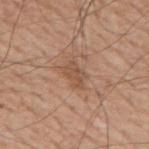Imaged during a routine full-body skin examination; the lesion was not biopsied and no histopathology is available. From the mid back. The tile uses white-light illumination. The lesion-visualizer software estimated a footprint of about 5 mm² and a shape eccentricity near 0.8. And it measured a classifier nevus-likeness of about 0/100 and a lesion-detection confidence of about 100/100. The patient is a male aged approximately 75. A region of skin cropped from a whole-body photographic capture, roughly 15 mm wide.